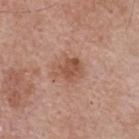Assessment:
Part of a total-body skin-imaging series; this lesion was reviewed on a skin check and was not flagged for biopsy.
Acquisition and patient details:
Automated image analysis of the tile measured an area of roughly 6.5 mm², a shape eccentricity near 0.65, and two-axis asymmetry of about 0.35. The analysis additionally found a lesion color around L≈52 a*≈23 b*≈30 in CIELAB, about 9 CIELAB-L* units darker than the surrounding skin, and a lesion-to-skin contrast of about 7 (normalized; higher = more distinct). The analysis additionally found a border-irregularity index near 4/10, internal color variation of about 2.5 on a 0–10 scale, and peripheral color asymmetry of about 1. On the upper back. Approximately 3.5 mm at its widest. A male subject aged approximately 65. The tile uses white-light illumination. Cropped from a whole-body photographic skin survey; the tile spans about 15 mm.